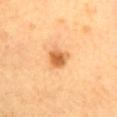Clinical impression:
Part of a total-body skin-imaging series; this lesion was reviewed on a skin check and was not flagged for biopsy.
Context:
A female patient roughly 45 years of age. On the mid back. Automated image analysis of the tile measured an eccentricity of roughly 0.6 and two-axis asymmetry of about 0.25. The software also gave a color-variation rating of about 4.5/10 and a peripheral color-asymmetry measure near 1.5. The tile uses cross-polarized illumination. The recorded lesion diameter is about 3 mm. A 15 mm close-up extracted from a 3D total-body photography capture.